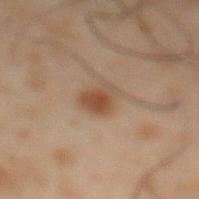<lesion>
  <biopsy_status>not biopsied; imaged during a skin examination</biopsy_status>
  <image>
    <source>total-body photography crop</source>
    <field_of_view_mm>15</field_of_view_mm>
  </image>
  <automated_metrics>
    <area_mm2_approx>6.0</area_mm2_approx>
    <eccentricity>0.7</eccentricity>
    <shape_asymmetry>0.35</shape_asymmetry>
    <cielab_L>36</cielab_L>
    <cielab_a>15</cielab_a>
    <cielab_b>24</cielab_b>
    <vs_skin_darker_L>8.0</vs_skin_darker_L>
    <vs_skin_contrast_norm>7.5</vs_skin_contrast_norm>
  </automated_metrics>
  <lesion_size>
    <long_diameter_mm_approx>3.0</long_diameter_mm_approx>
  </lesion_size>
  <patient>
    <sex>male</sex>
    <age_approx>50</age_approx>
  </patient>
  <site>abdomen</site>
</lesion>